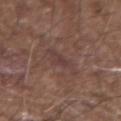  biopsy_status: not biopsied; imaged during a skin examination
  image:
    source: total-body photography crop
    field_of_view_mm: 15
  site: arm
  lighting: white-light
  patient:
    sex: male
    age_approx: 75